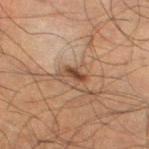Captured under cross-polarized illumination.
A 15 mm close-up extracted from a 3D total-body photography capture.
The lesion is on the leg.
A male patient in their 50s.
Approximately 2.5 mm at its widest.